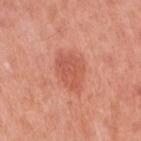Recorded during total-body skin imaging; not selected for excision or biopsy.
This image is a 15 mm lesion crop taken from a total-body photograph.
A female subject, approximately 50 years of age.
The lesion's longest dimension is about 4.5 mm.
The total-body-photography lesion software estimated an average lesion color of about L≈57 a*≈30 b*≈33 (CIELAB) and a normalized lesion–skin contrast near 6. The analysis additionally found a border-irregularity rating of about 2.5/10, internal color variation of about 3 on a 0–10 scale, and a peripheral color-asymmetry measure near 1.
The lesion is on the right upper arm.
Imaged with white-light lighting.